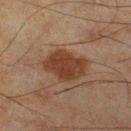Clinical impression:
No biopsy was performed on this lesion — it was imaged during a full skin examination and was not determined to be concerning.
Clinical summary:
Located on the right lower leg. A male subject aged around 45. A close-up tile cropped from a whole-body skin photograph, about 15 mm across.